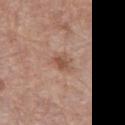biopsy status = no biopsy performed (imaged during a skin exam)
tile lighting = white-light illumination
anatomic site = the right thigh
acquisition = ~15 mm tile from a whole-body skin photo
lesion size = ~2.5 mm (longest diameter)
patient = female, roughly 60 years of age
automated metrics = a footprint of about 4.5 mm² and an eccentricity of roughly 0.5; a mean CIELAB color near L≈53 a*≈20 b*≈29, roughly 9 lightness units darker than nearby skin, and a normalized lesion–skin contrast near 6.5; internal color variation of about 3 on a 0–10 scale